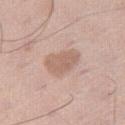follow-up: imaged on a skin check; not biopsied | location: the right thigh | imaging modality: total-body-photography crop, ~15 mm field of view | subject: male, in their 60s.